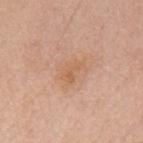Image and clinical context: From the mid back. This image is a 15 mm lesion crop taken from a total-body photograph. Automated tile analysis of the lesion measured an area of roughly 4.5 mm² and an eccentricity of roughly 0.75. And it measured a lesion–skin lightness drop of about 6. And it measured border irregularity of about 3.5 on a 0–10 scale, internal color variation of about 2.5 on a 0–10 scale, and a peripheral color-asymmetry measure near 1. A male patient aged around 70.